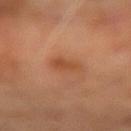Image and clinical context:
The subject is a male approximately 70 years of age. This image is a 15 mm lesion crop taken from a total-body photograph. The lesion is located on the left forearm.